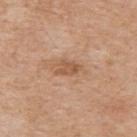- follow-up · no biopsy performed (imaged during a skin exam)
- patient · male, approximately 60 years of age
- illumination · white-light illumination
- acquisition · 15 mm crop, total-body photography
- location · the upper back